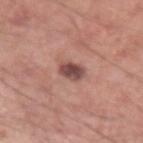  biopsy_status: not biopsied; imaged during a skin examination
  lesion_size:
    long_diameter_mm_approx: 3.0
  site: left upper arm
  patient:
    sex: male
    age_approx: 60
  image:
    source: total-body photography crop
    field_of_view_mm: 15